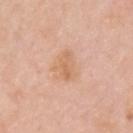Notes:
• image source — ~15 mm tile from a whole-body skin photo
• TBP lesion metrics — internal color variation of about 3 on a 0–10 scale and peripheral color asymmetry of about 1
• anatomic site — the upper back
• size — ~3 mm (longest diameter)
• subject — male, in their 60s
• tile lighting — white-light illumination